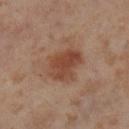Q: Was a biopsy performed?
A: catalogued during a skin exam; not biopsied
Q: How was this image acquired?
A: 15 mm crop, total-body photography
Q: Where on the body is the lesion?
A: the left lower leg
Q: Patient demographics?
A: female, roughly 55 years of age
Q: Illumination type?
A: cross-polarized illumination
Q: What is the lesion's diameter?
A: ~4.5 mm (longest diameter)